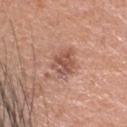Q: Was this lesion biopsied?
A: total-body-photography surveillance lesion; no biopsy
Q: What is the imaging modality?
A: ~15 mm crop, total-body skin-cancer survey
Q: Lesion location?
A: the head or neck
Q: Patient demographics?
A: male, aged 53 to 57
Q: Automated lesion metrics?
A: a nevus-likeness score of about 10/100
Q: What lighting was used for the tile?
A: white-light illumination
Q: Lesion size?
A: about 3.5 mm